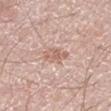Notes:
- follow-up — total-body-photography surveillance lesion; no biopsy
- image source — 15 mm crop, total-body photography
- body site — the leg
- subject — male, in their 70s
- size — about 3 mm
- illumination — white-light